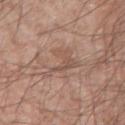workup = imaged on a skin check; not biopsied
site = the left forearm
illumination = white-light
image source = ~15 mm tile from a whole-body skin photo
subject = male, approximately 60 years of age